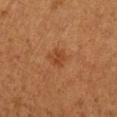follow-up: imaged on a skin check; not biopsied
lighting: cross-polarized illumination
subject: female, approximately 40 years of age
automated metrics: a within-lesion color-variation index near 2.5/10; a classifier nevus-likeness of about 45/100
anatomic site: the arm
image source: ~15 mm crop, total-body skin-cancer survey